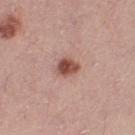<record>
<biopsy_status>not biopsied; imaged during a skin examination</biopsy_status>
<site>left thigh</site>
<lesion_size>
  <long_diameter_mm_approx>2.5</long_diameter_mm_approx>
</lesion_size>
<image>
  <source>total-body photography crop</source>
  <field_of_view_mm>15</field_of_view_mm>
</image>
<patient>
  <sex>female</sex>
  <age_approx>40</age_approx>
</patient>
<lighting>white-light</lighting>
</record>